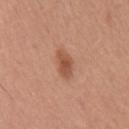Imaged during a routine full-body skin examination; the lesion was not biopsied and no histopathology is available.
A 15 mm crop from a total-body photograph taken for skin-cancer surveillance.
This is a white-light tile.
Located on the upper back.
A male patient aged 53–57.